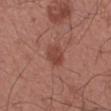automated metrics=a mean CIELAB color near L≈44 a*≈25 b*≈27; border irregularity of about 1 on a 0–10 scale and peripheral color asymmetry of about 1; a classifier nevus-likeness of about 50/100 and a detector confidence of about 100 out of 100 that the crop contains a lesion
lesion size=≈2.5 mm
image source=15 mm crop, total-body photography
lighting=white-light illumination
patient=male, in their mid- to late 30s
anatomic site=the left forearm